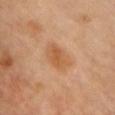notes = no biopsy performed (imaged during a skin exam)
size = about 4 mm
automated metrics = a lesion area of about 8 mm², an eccentricity of roughly 0.8, and a symmetry-axis asymmetry near 0.25; an average lesion color of about L≈59 a*≈23 b*≈39 (CIELAB), a lesion–skin lightness drop of about 8, and a normalized lesion–skin contrast near 6.5; a color-variation rating of about 3/10
acquisition = ~15 mm crop, total-body skin-cancer survey
tile lighting = cross-polarized illumination
body site = the front of the torso
subject = female, aged around 40